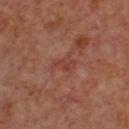Captured during whole-body skin photography for melanoma surveillance; the lesion was not biopsied.
Imaged with cross-polarized lighting.
The total-body-photography lesion software estimated a footprint of about 3.5 mm², a shape eccentricity near 0.85, and two-axis asymmetry of about 0.35. It also reported a normalized lesion–skin contrast near 5.5.
A male subject about 65 years old.
Cropped from a total-body skin-imaging series; the visible field is about 15 mm.
The recorded lesion diameter is about 3 mm.